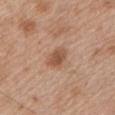follow-up: imaged on a skin check; not biopsied | size: about 3 mm | image: ~15 mm tile from a whole-body skin photo | body site: the left upper arm | lighting: white-light illumination | patient: male, aged 63 to 67.